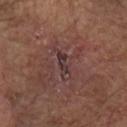This lesion was catalogued during total-body skin photography and was not selected for biopsy.
The lesion is on the head or neck.
Captured under white-light illumination.
Measured at roughly 4.5 mm in maximum diameter.
A male patient aged around 80.
A 15 mm close-up extracted from a 3D total-body photography capture.